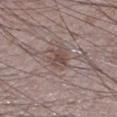Q: Is there a histopathology result?
A: catalogued during a skin exam; not biopsied
Q: How was this image acquired?
A: total-body-photography crop, ~15 mm field of view
Q: Where on the body is the lesion?
A: the leg
Q: Patient demographics?
A: male, approximately 75 years of age
Q: Automated lesion metrics?
A: a classifier nevus-likeness of about 5/100 and a lesion-detection confidence of about 95/100
Q: What lighting was used for the tile?
A: white-light illumination